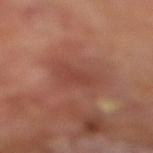<record>
  <biopsy_status>not biopsied; imaged during a skin examination</biopsy_status>
  <image>
    <source>total-body photography crop</source>
    <field_of_view_mm>15</field_of_view_mm>
  </image>
  <automated_metrics>
    <cielab_L>43</cielab_L>
    <cielab_a>25</cielab_a>
    <cielab_b>27</cielab_b>
    <vs_skin_darker_L>6.0</vs_skin_darker_L>
    <border_irregularity_0_10>3.0</border_irregularity_0_10>
    <color_variation_0_10>2.5</color_variation_0_10>
    <peripheral_color_asymmetry>1.0</peripheral_color_asymmetry>
    <nevus_likeness_0_100>0</nevus_likeness_0_100>
    <lesion_detection_confidence_0_100>100</lesion_detection_confidence_0_100>
  </automated_metrics>
  <lighting>cross-polarized</lighting>
  <site>right lower leg</site>
  <patient>
    <sex>male</sex>
    <age_approx>70</age_approx>
  </patient>
</record>